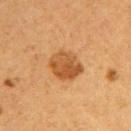{"image": {"source": "total-body photography crop", "field_of_view_mm": 15}, "site": "right upper arm", "lighting": "cross-polarized", "patient": {"sex": "female", "age_approx": 40}, "lesion_size": {"long_diameter_mm_approx": 3.5}, "automated_metrics": {"cielab_L": 44, "cielab_a": 21, "cielab_b": 36, "vs_skin_darker_L": 10.0, "nevus_likeness_0_100": 65}}